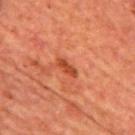Findings:
– notes · total-body-photography surveillance lesion; no biopsy
– subject · male, in their mid- to late 60s
– illumination · cross-polarized
– acquisition · 15 mm crop, total-body photography
– TBP lesion metrics · an eccentricity of roughly 0.9; a border-irregularity index near 3.5/10
– body site · the back